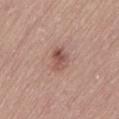Assessment: This lesion was catalogued during total-body skin photography and was not selected for biopsy.